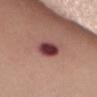Recorded during total-body skin imaging; not selected for excision or biopsy.
A 15 mm crop from a total-body photograph taken for skin-cancer surveillance.
The lesion is located on the abdomen.
This is a white-light tile.
A female subject, aged around 70.
Longest diameter approximately 4 mm.